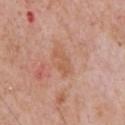Q: Was a biopsy performed?
A: catalogued during a skin exam; not biopsied
Q: Lesion size?
A: about 3.5 mm
Q: What is the anatomic site?
A: the chest
Q: Who is the patient?
A: male, aged approximately 60
Q: What lighting was used for the tile?
A: white-light illumination
Q: What kind of image is this?
A: 15 mm crop, total-body photography